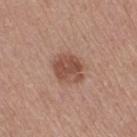{"lighting": "white-light", "image": {"source": "total-body photography crop", "field_of_view_mm": 15}, "automated_metrics": {"nevus_likeness_0_100": 50, "lesion_detection_confidence_0_100": 100}, "patient": {"sex": "female", "age_approx": 55}, "site": "leg"}